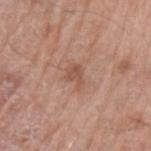| field | value |
|---|---|
| workup | catalogued during a skin exam; not biopsied |
| patient | male, roughly 65 years of age |
| body site | the left upper arm |
| automated metrics | an area of roughly 4 mm², an eccentricity of roughly 0.75, and a symmetry-axis asymmetry near 0.4 |
| lesion size | ≈3 mm |
| imaging modality | ~15 mm crop, total-body skin-cancer survey |
| lighting | white-light |